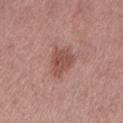The lesion was photographed on a routine skin check and not biopsied; there is no pathology result.
Longest diameter approximately 4 mm.
The lesion is on the left lower leg.
The tile uses white-light illumination.
An algorithmic analysis of the crop reported an area of roughly 8 mm², an outline eccentricity of about 0.7 (0 = round, 1 = elongated), and a shape-asymmetry score of about 0.3 (0 = symmetric). It also reported a border-irregularity rating of about 2.5/10, a within-lesion color-variation index near 2.5/10, and radial color variation of about 1. The software also gave a classifier nevus-likeness of about 30/100 and a lesion-detection confidence of about 100/100.
A region of skin cropped from a whole-body photographic capture, roughly 15 mm wide.
A female patient, aged around 40.